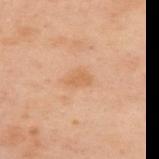Findings:
* workup · total-body-photography surveillance lesion; no biopsy
* tile lighting · cross-polarized illumination
* subject · female, aged around 55
* automated metrics · a detector confidence of about 100 out of 100 that the crop contains a lesion
* lesion diameter · about 2.5 mm
* acquisition · ~15 mm crop, total-body skin-cancer survey
* site · the upper back